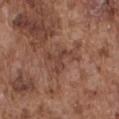<record>
  <biopsy_status>not biopsied; imaged during a skin examination</biopsy_status>
  <lesion_size>
    <long_diameter_mm_approx>5.5</long_diameter_mm_approx>
  </lesion_size>
  <image>
    <source>total-body photography crop</source>
    <field_of_view_mm>15</field_of_view_mm>
  </image>
  <lighting>white-light</lighting>
  <site>chest</site>
  <patient>
    <sex>male</sex>
    <age_approx>75</age_approx>
  </patient>
</record>